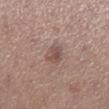The lesion is located on the leg. Automated tile analysis of the lesion measured a footprint of about 5.5 mm², an outline eccentricity of about 0.8 (0 = round, 1 = elongated), and a shape-asymmetry score of about 0.25 (0 = symmetric). It also reported a within-lesion color-variation index near 3.5/10 and radial color variation of about 1. It also reported an automated nevus-likeness rating near 0 out of 100 and a detector confidence of about 100 out of 100 that the crop contains a lesion. The patient is a female roughly 40 years of age. This is a white-light tile. The lesion's longest dimension is about 3.5 mm. A lesion tile, about 15 mm wide, cut from a 3D total-body photograph.